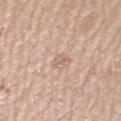Imaged during a routine full-body skin examination; the lesion was not biopsied and no histopathology is available.
Imaged with white-light lighting.
Located on the left upper arm.
Longest diameter approximately 2.5 mm.
The subject is a male roughly 80 years of age.
A close-up tile cropped from a whole-body skin photograph, about 15 mm across.